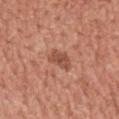The lesion is on the chest. Captured under white-light illumination. Approximately 3.5 mm at its widest. The lesion-visualizer software estimated a within-lesion color-variation index near 2.5/10. A 15 mm close-up tile from a total-body photography series done for melanoma screening. A male patient, aged 43 to 47.